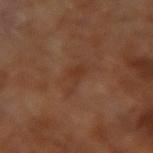Recorded during total-body skin imaging; not selected for excision or biopsy. A male subject aged 63–67. A region of skin cropped from a whole-body photographic capture, roughly 15 mm wide. Longest diameter approximately 3.5 mm.